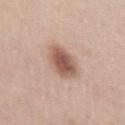Notes:
* workup — total-body-photography surveillance lesion; no biopsy
* illumination — white-light
* patient — male, in their 60s
* acquisition — 15 mm crop, total-body photography
* site — the left upper arm
* image-analysis metrics — an automated nevus-likeness rating near 95 out of 100
* size — ≈4 mm